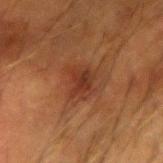  biopsy_status: not biopsied; imaged during a skin examination
  lesion_size:
    long_diameter_mm_approx: 3.0
  patient:
    sex: male
    age_approx: 65
  image:
    source: total-body photography crop
    field_of_view_mm: 15
  lighting: cross-polarized
  site: arm